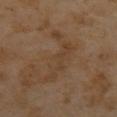Part of a total-body skin-imaging series; this lesion was reviewed on a skin check and was not flagged for biopsy.
A female subject, aged 53–57.
A 15 mm close-up tile from a total-body photography series done for melanoma screening.
Located on the mid back.